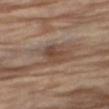The lesion was tiled from a total-body skin photograph and was not biopsied.
The lesion-visualizer software estimated a mean CIELAB color near L≈45 a*≈17 b*≈27 and a normalized lesion–skin contrast near 7.5. It also reported border irregularity of about 2.5 on a 0–10 scale and a within-lesion color-variation index near 5/10.
On the right thigh.
The recorded lesion diameter is about 3 mm.
Cropped from a whole-body photographic skin survey; the tile spans about 15 mm.
A male subject approximately 70 years of age.